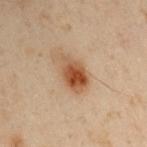biopsy status = total-body-photography surveillance lesion; no biopsy
imaging modality = ~15 mm tile from a whole-body skin photo
TBP lesion metrics = an eccentricity of roughly 0.8; a peripheral color-asymmetry measure near 2.5
anatomic site = the left arm
patient = male, about 50 years old
lesion diameter = about 4.5 mm